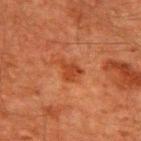Findings:
* biopsy status: total-body-photography surveillance lesion; no biopsy
* diameter: ≈3 mm
* lighting: cross-polarized illumination
* subject: male, approximately 60 years of age
* image: 15 mm crop, total-body photography
* anatomic site: the upper back